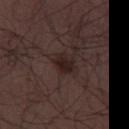Recorded during total-body skin imaging; not selected for excision or biopsy. A male subject, about 30 years old. The lesion's longest dimension is about 2.5 mm. The tile uses white-light illumination. On the abdomen. A lesion tile, about 15 mm wide, cut from a 3D total-body photograph.